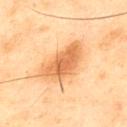Assessment:
This lesion was catalogued during total-body skin photography and was not selected for biopsy.
Image and clinical context:
About 6 mm across. The subject is a male roughly 45 years of age. Located on the upper back. The tile uses cross-polarized illumination. This image is a 15 mm lesion crop taken from a total-body photograph.